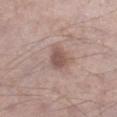Impression:
Imaged during a routine full-body skin examination; the lesion was not biopsied and no histopathology is available.
Clinical summary:
A region of skin cropped from a whole-body photographic capture, roughly 15 mm wide. The lesion is located on the right lower leg. Approximately 3 mm at its widest. This is a white-light tile. A male patient aged 53–57. The total-body-photography lesion software estimated internal color variation of about 2 on a 0–10 scale and radial color variation of about 0.5. The analysis additionally found an automated nevus-likeness rating near 30 out of 100 and a detector confidence of about 100 out of 100 that the crop contains a lesion.